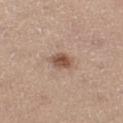Clinical impression:
Captured during whole-body skin photography for melanoma surveillance; the lesion was not biopsied.
Image and clinical context:
From the leg. Longest diameter approximately 2.5 mm. This image is a 15 mm lesion crop taken from a total-body photograph. A female subject about 45 years old.